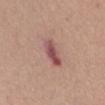| field | value |
|---|---|
| biopsy status | total-body-photography surveillance lesion; no biopsy |
| patient | female, aged approximately 50 |
| site | the front of the torso |
| imaging modality | ~15 mm tile from a whole-body skin photo |
| TBP lesion metrics | a footprint of about 7 mm² and two-axis asymmetry of about 0.15; roughly 12 lightness units darker than nearby skin |